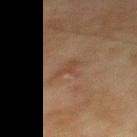Q: Was a biopsy performed?
A: catalogued during a skin exam; not biopsied
Q: What is the lesion's diameter?
A: ~2.5 mm (longest diameter)
Q: Automated lesion metrics?
A: lesion-presence confidence of about 100/100
Q: Where on the body is the lesion?
A: the upper back
Q: What are the patient's age and sex?
A: male, aged 73–77
Q: How was this image acquired?
A: ~15 mm crop, total-body skin-cancer survey